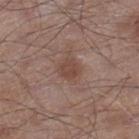Assessment:
Imaged during a routine full-body skin examination; the lesion was not biopsied and no histopathology is available.
Background:
A male patient, approximately 55 years of age. The lesion is on the right lower leg. Imaged with white-light lighting. A region of skin cropped from a whole-body photographic capture, roughly 15 mm wide.